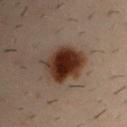Impression: Part of a total-body skin-imaging series; this lesion was reviewed on a skin check and was not flagged for biopsy. Clinical summary: The subject is a male aged around 30. A roughly 15 mm field-of-view crop from a total-body skin photograph. The lesion is located on the left upper arm. The lesion's longest dimension is about 5 mm.